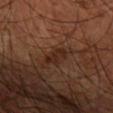Q: Was this lesion biopsied?
A: imaged on a skin check; not biopsied
Q: Lesion size?
A: ~3.5 mm (longest diameter)
Q: How was this image acquired?
A: 15 mm crop, total-body photography
Q: Patient demographics?
A: male, aged approximately 60
Q: Lesion location?
A: the right forearm
Q: How was the tile lit?
A: cross-polarized